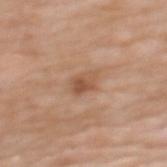follow-up = imaged on a skin check; not biopsied
patient = female, in their 70s
location = the upper back
image = 15 mm crop, total-body photography